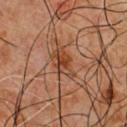{
  "site": "chest",
  "image": {
    "source": "total-body photography crop",
    "field_of_view_mm": 15
  },
  "patient": {
    "sex": "male",
    "age_approx": 60
  }
}